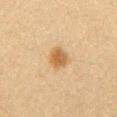Assessment: This lesion was catalogued during total-body skin photography and was not selected for biopsy. Image and clinical context: From the chest. A male patient, roughly 35 years of age. A 15 mm close-up tile from a total-body photography series done for melanoma screening. The recorded lesion diameter is about 3 mm. The tile uses cross-polarized illumination. The lesion-visualizer software estimated a footprint of about 6 mm², an eccentricity of roughly 0.6, and a symmetry-axis asymmetry near 0.2. The analysis additionally found about 9 CIELAB-L* units darker than the surrounding skin and a normalized border contrast of about 8. The software also gave a border-irregularity index near 2/10, a color-variation rating of about 2.5/10, and a peripheral color-asymmetry measure near 0.5. The analysis additionally found an automated nevus-likeness rating near 100 out of 100 and lesion-presence confidence of about 100/100.